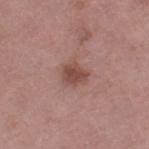About 3 mm across. Automated image analysis of the tile measured a shape eccentricity near 0.55. And it measured a mean CIELAB color near L≈47 a*≈23 b*≈24, roughly 10 lightness units darker than nearby skin, and a normalized lesion–skin contrast near 8. It also reported a border-irregularity rating of about 2.5/10, internal color variation of about 2.5 on a 0–10 scale, and peripheral color asymmetry of about 1. The analysis additionally found a classifier nevus-likeness of about 50/100 and a detector confidence of about 100 out of 100 that the crop contains a lesion. A female subject, aged around 70. Located on the leg. Cropped from a total-body skin-imaging series; the visible field is about 15 mm.